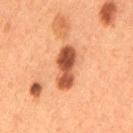Clinical impression: This lesion was catalogued during total-body skin photography and was not selected for biopsy. Acquisition and patient details: The tile uses cross-polarized illumination. A 15 mm close-up tile from a total-body photography series done for melanoma screening. An algorithmic analysis of the crop reported an area of roughly 11 mm² and an eccentricity of roughly 0.9. The software also gave peripheral color asymmetry of about 2.5. And it measured a nevus-likeness score of about 90/100 and a lesion-detection confidence of about 100/100. Longest diameter approximately 5.5 mm. A female patient, approximately 50 years of age. On the left thigh.